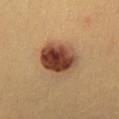biopsy status — imaged on a skin check; not biopsied | anatomic site — the chest | imaging modality — ~15 mm tile from a whole-body skin photo | tile lighting — cross-polarized illumination | diameter — ~5 mm (longest diameter) | automated metrics — a footprint of about 17 mm² and two-axis asymmetry of about 0.15; a lesion–skin lightness drop of about 18 and a lesion-to-skin contrast of about 13.5 (normalized; higher = more distinct); an automated nevus-likeness rating near 100 out of 100 and a lesion-detection confidence of about 100/100 | patient — male, aged 38–42.